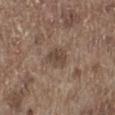Notes:
• diameter · about 3 mm
• subject · male, in their 70s
• body site · the left lower leg
• image source · total-body-photography crop, ~15 mm field of view